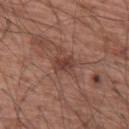The lesion was photographed on a routine skin check and not biopsied; there is no pathology result.
On the right upper arm.
Approximately 3 mm at its widest.
Captured under white-light illumination.
A male subject aged 63 to 67.
The total-body-photography lesion software estimated a footprint of about 4.5 mm², an eccentricity of roughly 0.8, and a symmetry-axis asymmetry near 0.2. The analysis additionally found a mean CIELAB color near L≈40 a*≈22 b*≈24, about 9 CIELAB-L* units darker than the surrounding skin, and a lesion-to-skin contrast of about 7.5 (normalized; higher = more distinct). And it measured a within-lesion color-variation index near 4/10 and radial color variation of about 1.5.
A region of skin cropped from a whole-body photographic capture, roughly 15 mm wide.